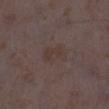No biopsy was performed on this lesion — it was imaged during a full skin examination and was not determined to be concerning. Captured under white-light illumination. A 15 mm close-up tile from a total-body photography series done for melanoma screening. A female patient about 35 years old. Located on the right lower leg. The total-body-photography lesion software estimated an automated nevus-likeness rating near 0 out of 100 and lesion-presence confidence of about 100/100.